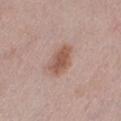Part of a total-body skin-imaging series; this lesion was reviewed on a skin check and was not flagged for biopsy. The tile uses white-light illumination. The total-body-photography lesion software estimated a mean CIELAB color near L≈55 a*≈20 b*≈26, roughly 10 lightness units darker than nearby skin, and a lesion-to-skin contrast of about 7.5 (normalized; higher = more distinct). Longest diameter approximately 4 mm. A female subject aged 28 to 32. On the left thigh. A 15 mm close-up extracted from a 3D total-body photography capture.